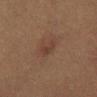Clinical impression:
Imaged during a routine full-body skin examination; the lesion was not biopsied and no histopathology is available.
Acquisition and patient details:
A lesion tile, about 15 mm wide, cut from a 3D total-body photograph. Imaged with cross-polarized lighting. A female subject, about 55 years old. Located on the abdomen.